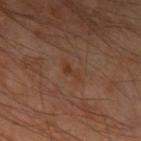Q: Patient demographics?
A: male, aged around 65
Q: Lesion size?
A: about 2.5 mm
Q: Lesion location?
A: the arm
Q: How was this image acquired?
A: ~15 mm tile from a whole-body skin photo
Q: Illumination type?
A: cross-polarized
Q: Automated lesion metrics?
A: an area of roughly 3 mm² and an outline eccentricity of about 0.85 (0 = round, 1 = elongated); a border-irregularity rating of about 4/10, internal color variation of about 0 on a 0–10 scale, and a peripheral color-asymmetry measure near 0